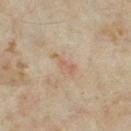{
  "biopsy_status": "not biopsied; imaged during a skin examination"
}